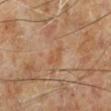Case summary:
• follow-up: total-body-photography surveillance lesion; no biopsy
• subject: male, roughly 70 years of age
• acquisition: total-body-photography crop, ~15 mm field of view
• location: the left lower leg